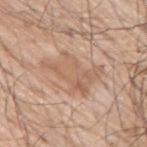This lesion was catalogued during total-body skin photography and was not selected for biopsy.
A male subject, aged 68 to 72.
A 15 mm close-up tile from a total-body photography series done for melanoma screening.
Captured under white-light illumination.
The lesion is on the upper back.